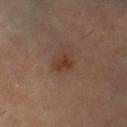Located on the leg.
The subject is a female in their 70s.
Cropped from a total-body skin-imaging series; the visible field is about 15 mm.